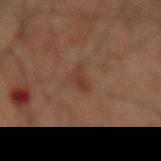biopsy status: imaged on a skin check; not biopsied
image: 15 mm crop, total-body photography
site: the mid back
patient: male, aged around 60
illumination: cross-polarized illumination
TBP lesion metrics: a lesion color around L≈33 a*≈17 b*≈26 in CIELAB